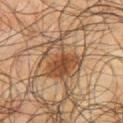Impression: The lesion was photographed on a routine skin check and not biopsied; there is no pathology result. Image and clinical context: The patient is a male about 65 years old. About 5.5 mm across. Located on the back. A region of skin cropped from a whole-body photographic capture, roughly 15 mm wide.